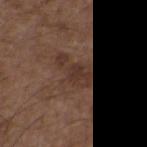Assessment:
Recorded during total-body skin imaging; not selected for excision or biopsy.
Background:
A 15 mm crop from a total-body photograph taken for skin-cancer surveillance. The lesion-visualizer software estimated a footprint of about 12 mm² and two-axis asymmetry of about 0.45. The software also gave a lesion color around L≈35 a*≈17 b*≈24 in CIELAB, a lesion–skin lightness drop of about 7, and a normalized lesion–skin contrast near 6.5. And it measured a nevus-likeness score of about 0/100 and lesion-presence confidence of about 60/100. The lesion's longest dimension is about 5.5 mm. Located on the chest. A male subject, roughly 50 years of age.